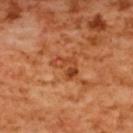This lesion was catalogued during total-body skin photography and was not selected for biopsy. A 15 mm crop from a total-body photograph taken for skin-cancer surveillance. The lesion is located on the upper back. The subject is a female aged around 55.